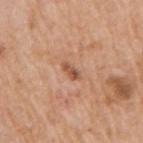Clinical impression: Captured during whole-body skin photography for melanoma surveillance; the lesion was not biopsied. Clinical summary: A 15 mm close-up extracted from a 3D total-body photography capture. The lesion is located on the right upper arm. A male patient about 60 years old.